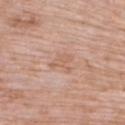Assessment:
This lesion was catalogued during total-body skin photography and was not selected for biopsy.
Acquisition and patient details:
A 15 mm close-up tile from a total-body photography series done for melanoma screening. An algorithmic analysis of the crop reported an automated nevus-likeness rating near 0 out of 100 and lesion-presence confidence of about 100/100. This is a white-light tile. Measured at roughly 3 mm in maximum diameter. A female subject, about 70 years old. The lesion is located on the upper back.